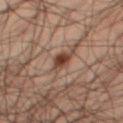Impression: Captured during whole-body skin photography for melanoma surveillance; the lesion was not biopsied. Context: A male subject, approximately 50 years of age. Imaged with cross-polarized lighting. The lesion is located on the leg. Measured at roughly 2.5 mm in maximum diameter. A 15 mm crop from a total-body photograph taken for skin-cancer surveillance.